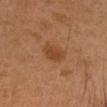body site = the head or neck; subject = female, approximately 40 years of age; imaging modality = total-body-photography crop, ~15 mm field of view; size = about 2.5 mm; tile lighting = cross-polarized.